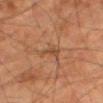notes: imaged on a skin check; not biopsied